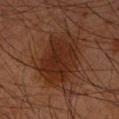Findings:
* workup — catalogued during a skin exam; not biopsied
* body site — the left forearm
* imaging modality — ~15 mm tile from a whole-body skin photo
* subject — male, approximately 60 years of age